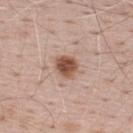Located on the upper back.
A male subject aged around 70.
A roughly 15 mm field-of-view crop from a total-body skin photograph.
This is a white-light tile.